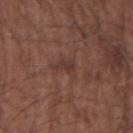workup — catalogued during a skin exam; not biopsied | lesion size — about 3.5 mm | lighting — white-light | image source — ~15 mm crop, total-body skin-cancer survey | patient — male, aged 63–67 | body site — the right forearm | TBP lesion metrics — a mean CIELAB color near L≈36 a*≈19 b*≈22.